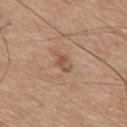<tbp_lesion>
<biopsy_status>not biopsied; imaged during a skin examination</biopsy_status>
<lighting>white-light</lighting>
<automated_metrics>
  <area_mm2_approx>3.5</area_mm2_approx>
  <shape_asymmetry>0.3</shape_asymmetry>
  <cielab_L>53</cielab_L>
  <cielab_a>19</cielab_a>
  <cielab_b>29</cielab_b>
  <vs_skin_darker_L>9.0</vs_skin_darker_L>
  <vs_skin_contrast_norm>6.0</vs_skin_contrast_norm>
  <color_variation_0_10>4.0</color_variation_0_10>
  <peripheral_color_asymmetry>1.5</peripheral_color_asymmetry>
</automated_metrics>
<site>mid back</site>
<patient>
  <sex>male</sex>
  <age_approx>75</age_approx>
</patient>
<image>
  <source>total-body photography crop</source>
  <field_of_view_mm>15</field_of_view_mm>
</image>
</tbp_lesion>